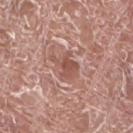The lesion is on the left lower leg.
This image is a 15 mm lesion crop taken from a total-body photograph.
A male subject aged around 75.
The lesion's longest dimension is about 3.5 mm.
Captured under white-light illumination.